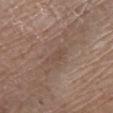Impression: Part of a total-body skin-imaging series; this lesion was reviewed on a skin check and was not flagged for biopsy. Acquisition and patient details: A region of skin cropped from a whole-body photographic capture, roughly 15 mm wide. A male patient aged 63–67. Longest diameter approximately 4 mm. The lesion is located on the left lower leg.